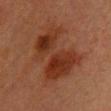Captured during whole-body skin photography for melanoma surveillance; the lesion was not biopsied. A female patient, approximately 40 years of age. Automated tile analysis of the lesion measured an area of roughly 26 mm², an outline eccentricity of about 0.75 (0 = round, 1 = elongated), and a symmetry-axis asymmetry near 0.35. It also reported an average lesion color of about L≈29 a*≈23 b*≈28 (CIELAB), about 8 CIELAB-L* units darker than the surrounding skin, and a normalized border contrast of about 8.5. The software also gave a border-irregularity rating of about 7/10 and internal color variation of about 5 on a 0–10 scale. The lesion is on the chest. This is a cross-polarized tile. A lesion tile, about 15 mm wide, cut from a 3D total-body photograph.